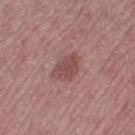workup = imaged on a skin check; not biopsied | site = the leg | image = 15 mm crop, total-body photography | subject = female, aged 43 to 47.